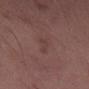Notes:
– biopsy status: catalogued during a skin exam; not biopsied
– acquisition: ~15 mm crop, total-body skin-cancer survey
– automated metrics: a lesion area of about 2.5 mm², a shape eccentricity near 0.95, and a symmetry-axis asymmetry near 0.55; an average lesion color of about L≈39 a*≈19 b*≈21 (CIELAB) and a normalized border contrast of about 4.5; a classifier nevus-likeness of about 0/100 and a detector confidence of about 80 out of 100 that the crop contains a lesion
– site: the left thigh
– lesion size: about 3.5 mm
– subject: male, aged approximately 50